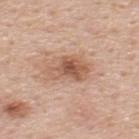notes: catalogued during a skin exam; not biopsied
anatomic site: the upper back
acquisition: ~15 mm tile from a whole-body skin photo
patient: male, aged 48–52
diameter: ≈5 mm
automated lesion analysis: a lesion area of about 11 mm²; about 11 CIELAB-L* units darker than the surrounding skin; a nevus-likeness score of about 70/100 and a lesion-detection confidence of about 100/100